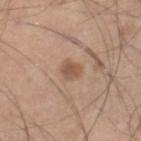Captured during whole-body skin photography for melanoma surveillance; the lesion was not biopsied.
The lesion is on the right lower leg.
A region of skin cropped from a whole-body photographic capture, roughly 15 mm wide.
The lesion's longest dimension is about 2.5 mm.
The patient is a male aged approximately 45.